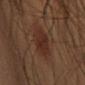Q: Was a biopsy performed?
A: no biopsy performed (imaged during a skin exam)
Q: What are the patient's age and sex?
A: male, in their mid-50s
Q: Lesion location?
A: the right upper arm
Q: How was the tile lit?
A: cross-polarized illumination
Q: What is the imaging modality?
A: ~15 mm tile from a whole-body skin photo
Q: What is the lesion's diameter?
A: ≈3.5 mm